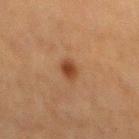Impression:
No biopsy was performed on this lesion — it was imaged during a full skin examination and was not determined to be concerning.
Context:
A male patient aged around 75. Imaged with cross-polarized lighting. Cropped from a total-body skin-imaging series; the visible field is about 15 mm. From the back. Approximately 2.5 mm at its widest. Automated image analysis of the tile measured a border-irregularity index near 2/10, a color-variation rating of about 1.5/10, and a peripheral color-asymmetry measure near 0.5.